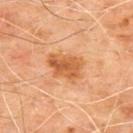Clinical impression:
Imaged during a routine full-body skin examination; the lesion was not biopsied and no histopathology is available.